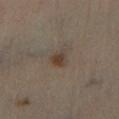Assessment: Captured during whole-body skin photography for melanoma surveillance; the lesion was not biopsied. Context: A 15 mm close-up extracted from a 3D total-body photography capture. Approximately 2 mm at its widest. Imaged with cross-polarized lighting. Located on the right lower leg. The subject is a male about 55 years old.